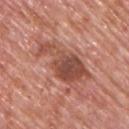Clinical impression:
No biopsy was performed on this lesion — it was imaged during a full skin examination and was not determined to be concerning.
Background:
Imaged with white-light lighting. A roughly 15 mm field-of-view crop from a total-body skin photograph. Measured at roughly 8 mm in maximum diameter. A male patient aged 73 to 77. The lesion is on the back.